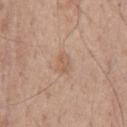Imaged during a routine full-body skin examination; the lesion was not biopsied and no histopathology is available. This is a white-light tile. A region of skin cropped from a whole-body photographic capture, roughly 15 mm wide. The lesion is on the chest. Longest diameter approximately 2.5 mm. A male patient about 55 years old.